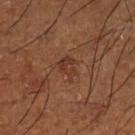Case summary:
- follow-up: total-body-photography surveillance lesion; no biopsy
- body site: the left lower leg
- image source: total-body-photography crop, ~15 mm field of view
- patient: male, in their mid- to late 60s